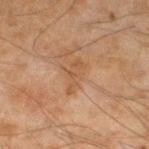| feature | finding |
|---|---|
| notes | catalogued during a skin exam; not biopsied |
| image | total-body-photography crop, ~15 mm field of view |
| subject | male, roughly 45 years of age |
| lesion diameter | ≈4 mm |
| location | the left lower leg |